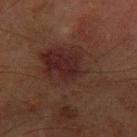  biopsy_status: not biopsied; imaged during a skin examination
  image:
    source: total-body photography crop
    field_of_view_mm: 15
  patient:
    sex: male
    age_approx: 65
  site: left forearm